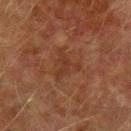Recorded during total-body skin imaging; not selected for excision or biopsy.
On the left upper arm.
This is a cross-polarized tile.
The lesion-visualizer software estimated an outline eccentricity of about 0.5 (0 = round, 1 = elongated) and a shape-asymmetry score of about 0.7 (0 = symmetric). The software also gave a lesion color around L≈29 a*≈19 b*≈26 in CIELAB, a lesion–skin lightness drop of about 5, and a normalized lesion–skin contrast near 5.5. It also reported a nevus-likeness score of about 0/100 and a lesion-detection confidence of about 100/100.
A roughly 15 mm field-of-view crop from a total-body skin photograph.
A male patient, aged approximately 75.